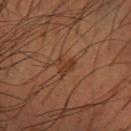The subject is a male aged approximately 60. This is a cross-polarized tile. Approximately 2.5 mm at its widest. A region of skin cropped from a whole-body photographic capture, roughly 15 mm wide. Located on the left forearm.